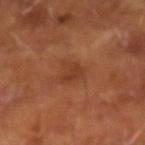Recorded during total-body skin imaging; not selected for excision or biopsy. A male patient, aged 63–67. Cropped from a total-body skin-imaging series; the visible field is about 15 mm. The tile uses cross-polarized illumination. The lesion's longest dimension is about 2.5 mm.